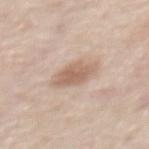No biopsy was performed on this lesion — it was imaged during a full skin examination and was not determined to be concerning. The tile uses white-light illumination. Located on the mid back. A male patient roughly 55 years of age. The total-body-photography lesion software estimated a nevus-likeness score of about 50/100. A close-up tile cropped from a whole-body skin photograph, about 15 mm across. The lesion's longest dimension is about 4 mm.